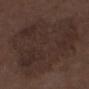{"patient": {"sex": "male", "age_approx": 75}, "lesion_size": {"long_diameter_mm_approx": 13.0}, "site": "left lower leg", "lighting": "white-light", "image": {"source": "total-body photography crop", "field_of_view_mm": 15}}